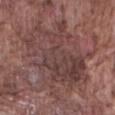workup — catalogued during a skin exam; not biopsied
illumination — white-light
patient — male, aged approximately 75
acquisition — ~15 mm crop, total-body skin-cancer survey
lesion diameter — ~10.5 mm (longest diameter)
site — the abdomen